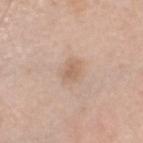<lesion>
  <biopsy_status>not biopsied; imaged during a skin examination</biopsy_status>
  <image>
    <source>total-body photography crop</source>
    <field_of_view_mm>15</field_of_view_mm>
  </image>
  <site>head or neck</site>
  <patient>
    <sex>male</sex>
    <age_approx>45</age_approx>
  </patient>
</lesion>